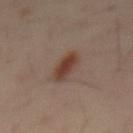Imaged during a routine full-body skin examination; the lesion was not biopsied and no histopathology is available.
A roughly 15 mm field-of-view crop from a total-body skin photograph.
The subject is a male aged around 40.
The lesion is on the mid back.
The recorded lesion diameter is about 4.5 mm.